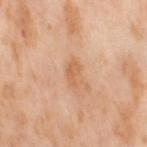Q: Was a biopsy performed?
A: total-body-photography surveillance lesion; no biopsy
Q: Who is the patient?
A: female, aged around 55
Q: Illumination type?
A: cross-polarized
Q: What kind of image is this?
A: ~15 mm tile from a whole-body skin photo
Q: How large is the lesion?
A: ~2.5 mm (longest diameter)
Q: Automated lesion metrics?
A: a footprint of about 3 mm², a shape eccentricity near 0.85, and a symmetry-axis asymmetry near 0.4; a detector confidence of about 100 out of 100 that the crop contains a lesion
Q: Where on the body is the lesion?
A: the right thigh